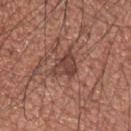workup: catalogued during a skin exam; not biopsied
lesion size: ≈4.5 mm
anatomic site: the upper back
image: total-body-photography crop, ~15 mm field of view
tile lighting: white-light
patient: male, aged approximately 60
automated lesion analysis: an area of roughly 7.5 mm², an eccentricity of roughly 0.75, and two-axis asymmetry of about 0.45; a mean CIELAB color near L≈43 a*≈21 b*≈25 and a normalized lesion–skin contrast near 7.5; lesion-presence confidence of about 80/100